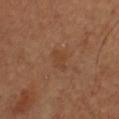Assessment: This lesion was catalogued during total-body skin photography and was not selected for biopsy. Acquisition and patient details: This image is a 15 mm lesion crop taken from a total-body photograph. A male subject, about 40 years old. The lesion is located on the chest.